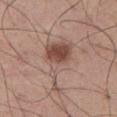biopsy_status: not biopsied; imaged during a skin examination
patient:
  sex: male
  age_approx: 65
lighting: white-light
image:
  source: total-body photography crop
  field_of_view_mm: 15
site: abdomen